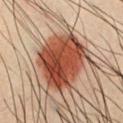Part of a total-body skin-imaging series; this lesion was reviewed on a skin check and was not flagged for biopsy. A male subject, aged around 35. The lesion is on the front of the torso. This is a cross-polarized tile. A 15 mm close-up tile from a total-body photography series done for melanoma screening. Approximately 6.5 mm at its widest.